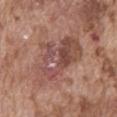The lesion was tiled from a total-body skin photograph and was not biopsied.
A 15 mm crop from a total-body photograph taken for skin-cancer surveillance.
The tile uses white-light illumination.
Located on the abdomen.
A male patient roughly 75 years of age.
The recorded lesion diameter is about 8 mm.